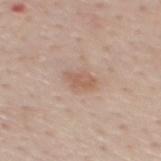anatomic site: the mid back
subject: male, aged around 55
lesion diameter: ≈3 mm
imaging modality: total-body-photography crop, ~15 mm field of view